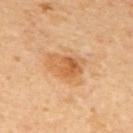Context: The subject is a male aged 58 to 62. Cropped from a total-body skin-imaging series; the visible field is about 15 mm. The lesion is on the mid back.